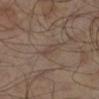Q: Was this lesion biopsied?
A: no biopsy performed (imaged during a skin exam)
Q: Lesion location?
A: the left lower leg
Q: Lesion size?
A: ≈2.5 mm
Q: What are the patient's age and sex?
A: male, aged around 65
Q: What kind of image is this?
A: ~15 mm tile from a whole-body skin photo
Q: What lighting was used for the tile?
A: cross-polarized illumination